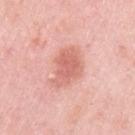Imaged during a routine full-body skin examination; the lesion was not biopsied and no histopathology is available. The tile uses white-light illumination. A female subject, about 65 years old. The recorded lesion diameter is about 5 mm. This image is a 15 mm lesion crop taken from a total-body photograph. Located on the left upper arm.